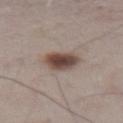workup: catalogued during a skin exam; not biopsied | automated lesion analysis: a lesion area of about 8.5 mm², a shape eccentricity near 0.55, and two-axis asymmetry of about 0.2; a mean CIELAB color near L≈45 a*≈16 b*≈21, about 15 CIELAB-L* units darker than the surrounding skin, and a normalized border contrast of about 11; a nevus-likeness score of about 100/100 | lighting: white-light illumination | patient: male, roughly 75 years of age | lesion size: ~3.5 mm (longest diameter) | site: the abdomen | image source: ~15 mm crop, total-body skin-cancer survey.